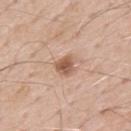The lesion was photographed on a routine skin check and not biopsied; there is no pathology result. On the upper back. Imaged with white-light lighting. The recorded lesion diameter is about 2.5 mm. A male patient, aged 63–67. This image is a 15 mm lesion crop taken from a total-body photograph.